Cropped from a whole-body photographic skin survey; the tile spans about 15 mm · on the left forearm · a female subject aged around 55 — 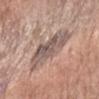Findings:
• tile lighting: white-light
• size: ~6.5 mm (longest diameter)
• histopathology: a lentigo (benign)